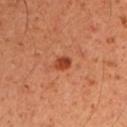follow-up — imaged on a skin check; not biopsied
subject — male, aged 48 to 52
acquisition — ~15 mm tile from a whole-body skin photo
body site — the left upper arm
automated metrics — an eccentricity of roughly 0.7 and two-axis asymmetry of about 0.15; a lesion color around L≈42 a*≈31 b*≈36 in CIELAB, a lesion–skin lightness drop of about 12, and a normalized border contrast of about 9; an automated nevus-likeness rating near 95 out of 100 and a lesion-detection confidence of about 100/100
lighting — cross-polarized
lesion size — ≈2 mm